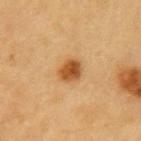biopsy_status: not biopsied; imaged during a skin examination
automated_metrics:
  cielab_L: 53
  cielab_a: 24
  cielab_b: 43
  vs_skin_darker_L: 14.0
  border_irregularity_0_10: 1.5
  color_variation_0_10: 4.5
  peripheral_color_asymmetry: 1.5
patient:
  sex: male
  age_approx: 60
lesion_size:
  long_diameter_mm_approx: 3.0
image:
  source: total-body photography crop
  field_of_view_mm: 15
site: left upper arm
lighting: cross-polarized